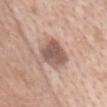biopsy_status: not biopsied; imaged during a skin examination
lighting: white-light
image:
  source: total-body photography crop
  field_of_view_mm: 15
lesion_size:
  long_diameter_mm_approx: 4.0
patient:
  sex: female
  age_approx: 75
site: head or neck